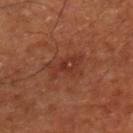Captured during whole-body skin photography for melanoma surveillance; the lesion was not biopsied. Located on the right lower leg. A subject aged approximately 65. Imaged with cross-polarized lighting. A region of skin cropped from a whole-body photographic capture, roughly 15 mm wide. Approximately 4.5 mm at its widest.